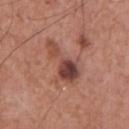{
  "biopsy_status": "not biopsied; imaged during a skin examination",
  "patient": {
    "sex": "male",
    "age_approx": 75
  },
  "lighting": "white-light",
  "image": {
    "source": "total-body photography crop",
    "field_of_view_mm": 15
  },
  "site": "chest",
  "lesion_size": {
    "long_diameter_mm_approx": 5.5
  }
}